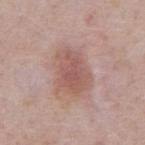Imaged during a routine full-body skin examination; the lesion was not biopsied and no histopathology is available. A male patient, about 75 years old. Automated tile analysis of the lesion measured a lesion area of about 14 mm² and two-axis asymmetry of about 0.2. The software also gave a mean CIELAB color near L≈55 a*≈21 b*≈24, roughly 9 lightness units darker than nearby skin, and a normalized border contrast of about 6.5. The lesion is located on the abdomen. A 15 mm crop from a total-body photograph taken for skin-cancer surveillance. This is a white-light tile. Longest diameter approximately 5 mm.